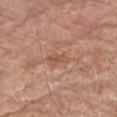Q: Is there a histopathology result?
A: catalogued during a skin exam; not biopsied
Q: How was this image acquired?
A: total-body-photography crop, ~15 mm field of view
Q: What lighting was used for the tile?
A: white-light
Q: Lesion location?
A: the right upper arm
Q: Lesion size?
A: ≈2.5 mm
Q: What are the patient's age and sex?
A: male, aged approximately 80
Q: Automated lesion metrics?
A: an outline eccentricity of about 0.9 (0 = round, 1 = elongated) and a symmetry-axis asymmetry near 0.35; a lesion color around L≈53 a*≈23 b*≈30 in CIELAB and a lesion–skin lightness drop of about 8